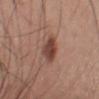<lesion>
  <biopsy_status>not biopsied; imaged during a skin examination</biopsy_status>
  <site>chest</site>
  <patient>
    <sex>male</sex>
    <age_approx>75</age_approx>
  </patient>
  <image>
    <source>total-body photography crop</source>
    <field_of_view_mm>15</field_of_view_mm>
  </image>
</lesion>